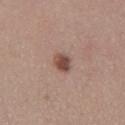notes: total-body-photography surveillance lesion; no biopsy | automated lesion analysis: a lesion area of about 4 mm², an eccentricity of roughly 0.7, and a shape-asymmetry score of about 0.15 (0 = symmetric); a border-irregularity rating of about 1.5/10; a nevus-likeness score of about 95/100 | image: total-body-photography crop, ~15 mm field of view | subject: male, roughly 55 years of age | illumination: white-light | site: the chest.